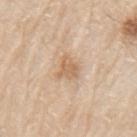Part of a total-body skin-imaging series; this lesion was reviewed on a skin check and was not flagged for biopsy.
A 15 mm crop from a total-body photograph taken for skin-cancer surveillance.
The lesion is located on the left upper arm.
A male subject aged 78 to 82.